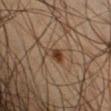Clinical impression: Captured during whole-body skin photography for melanoma surveillance; the lesion was not biopsied. Acquisition and patient details: Cropped from a whole-body photographic skin survey; the tile spans about 15 mm. A male patient, about 50 years old. The tile uses cross-polarized illumination. From the right forearm. The lesion's longest dimension is about 2.5 mm.